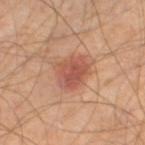Longest diameter approximately 4 mm. Captured under cross-polarized illumination. The patient is a male aged approximately 45. Cropped from a total-body skin-imaging series; the visible field is about 15 mm. Located on the left lower leg.